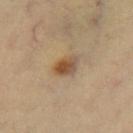Q: Was this lesion biopsied?
A: total-body-photography surveillance lesion; no biopsy
Q: How was this image acquired?
A: ~15 mm tile from a whole-body skin photo
Q: What is the anatomic site?
A: the leg
Q: Patient demographics?
A: female, aged 48–52